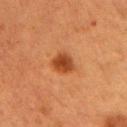The lesion was tiled from a total-body skin photograph and was not biopsied.
A close-up tile cropped from a whole-body skin photograph, about 15 mm across.
A female patient roughly 40 years of age.
About 3 mm across.
The lesion is on the left upper arm.
Automated tile analysis of the lesion measured a border-irregularity index near 2/10, internal color variation of about 2.5 on a 0–10 scale, and radial color variation of about 0.5.